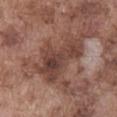biopsy_status: not biopsied; imaged during a skin examination
site: abdomen
patient:
  sex: male
  age_approx: 75
image:
  source: total-body photography crop
  field_of_view_mm: 15